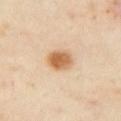workup: total-body-photography surveillance lesion; no biopsy
image: total-body-photography crop, ~15 mm field of view
TBP lesion metrics: a border-irregularity rating of about 1.5/10 and a color-variation rating of about 4.5/10; a nevus-likeness score of about 100/100 and lesion-presence confidence of about 100/100
location: the left upper arm
lesion size: ≈3 mm
lighting: cross-polarized illumination
patient: female, about 40 years old